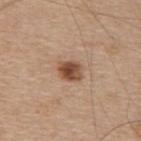Background:
This image is a 15 mm lesion crop taken from a total-body photograph. Located on the upper back. The patient is a male in their mid- to late 60s.